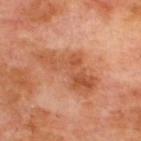<tbp_lesion>
<biopsy_status>not biopsied; imaged during a skin examination</biopsy_status>
<image>
  <source>total-body photography crop</source>
  <field_of_view_mm>15</field_of_view_mm>
</image>
<lesion_size>
  <long_diameter_mm_approx>7.5</long_diameter_mm_approx>
</lesion_size>
<lighting>cross-polarized</lighting>
<patient>
  <sex>male</sex>
  <age_approx>70</age_approx>
</patient>
<site>upper back</site>
</tbp_lesion>